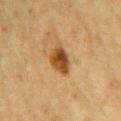Part of a total-body skin-imaging series; this lesion was reviewed on a skin check and was not flagged for biopsy.